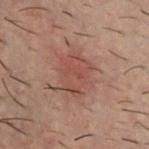This is a cross-polarized tile. The patient is a male aged around 30. A 15 mm crop from a total-body photograph taken for skin-cancer surveillance. The lesion is on the front of the torso. Automated tile analysis of the lesion measured an area of roughly 17 mm², a shape eccentricity near 0.7, and a shape-asymmetry score of about 0.2 (0 = symmetric). The analysis additionally found a mean CIELAB color near L≈39 a*≈18 b*≈21, a lesion–skin lightness drop of about 6, and a lesion-to-skin contrast of about 5.5 (normalized; higher = more distinct). And it measured border irregularity of about 3 on a 0–10 scale, internal color variation of about 3 on a 0–10 scale, and radial color variation of about 1.